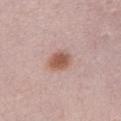| key | value |
|---|---|
| follow-up | imaged on a skin check; not biopsied |
| acquisition | 15 mm crop, total-body photography |
| automated metrics | a shape eccentricity near 0.65; a lesion color around L≈56 a*≈21 b*≈27 in CIELAB and a normalized border contrast of about 9 |
| tile lighting | white-light illumination |
| anatomic site | the chest |
| lesion size | about 3 mm |
| subject | female, aged 63 to 67 |